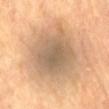Clinical impression: Part of a total-body skin-imaging series; this lesion was reviewed on a skin check and was not flagged for biopsy. Clinical summary: The tile uses cross-polarized illumination. A male subject, roughly 85 years of age or older. The total-body-photography lesion software estimated a lesion area of about 28 mm² and an eccentricity of roughly 0.35. Measured at roughly 6.5 mm in maximum diameter. Cropped from a total-body skin-imaging series; the visible field is about 15 mm. The lesion is on the chest.